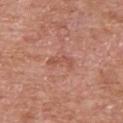The lesion was photographed on a routine skin check and not biopsied; there is no pathology result.
The lesion is located on the upper back.
A 15 mm crop from a total-body photograph taken for skin-cancer surveillance.
The subject is a male approximately 75 years of age.
Measured at roughly 3 mm in maximum diameter.